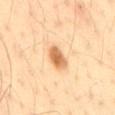Captured during whole-body skin photography for melanoma surveillance; the lesion was not biopsied. A 15 mm crop from a total-body photograph taken for skin-cancer surveillance. The subject is a male aged 33 to 37. The lesion is located on the lower back.